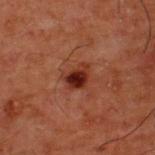The lesion was photographed on a routine skin check and not biopsied; there is no pathology result.